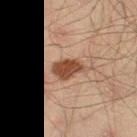Impression: The lesion was tiled from a total-body skin photograph and was not biopsied. Acquisition and patient details: The recorded lesion diameter is about 4 mm. A male subject aged 43–47. Cropped from a whole-body photographic skin survey; the tile spans about 15 mm. On the right thigh. This is a cross-polarized tile.